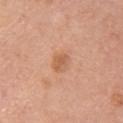biopsy_status: not biopsied; imaged during a skin examination
site: arm
patient:
  sex: female
  age_approx: 65
lighting: white-light
image:
  source: total-body photography crop
  field_of_view_mm: 15
lesion_size:
  long_diameter_mm_approx: 3.0